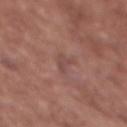* workup · no biopsy performed (imaged during a skin exam)
* lesion diameter · ~3 mm (longest diameter)
* tile lighting · white-light
* location · the chest
* subject · female, aged around 75
* imaging modality · ~15 mm tile from a whole-body skin photo